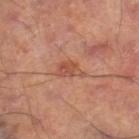Part of a total-body skin-imaging series; this lesion was reviewed on a skin check and was not flagged for biopsy.
From the right thigh.
The tile uses cross-polarized illumination.
About 3.5 mm across.
Cropped from a whole-body photographic skin survey; the tile spans about 15 mm.